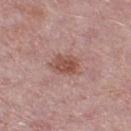{"biopsy_status": "not biopsied; imaged during a skin examination", "image": {"source": "total-body photography crop", "field_of_view_mm": 15}, "patient": {"sex": "female", "age_approx": 50}, "site": "leg", "automated_metrics": {"area_mm2_approx": 7.5, "shape_asymmetry": 0.15, "cielab_L": 51, "cielab_a": 23, "cielab_b": 25, "vs_skin_darker_L": 10.0}, "lesion_size": {"long_diameter_mm_approx": 3.5}, "lighting": "white-light"}